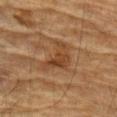Q: Was this lesion biopsied?
A: no biopsy performed (imaged during a skin exam)
Q: How was this image acquired?
A: ~15 mm tile from a whole-body skin photo
Q: Illumination type?
A: cross-polarized
Q: What are the patient's age and sex?
A: male, aged around 85 or older
Q: Where on the body is the lesion?
A: the left forearm
Q: What did automated image analysis measure?
A: a within-lesion color-variation index near 2.5/10 and radial color variation of about 1